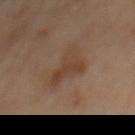lighting: cross-polarized
image: total-body-photography crop, ~15 mm field of view
site: the mid back